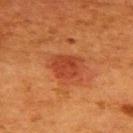The lesion was tiled from a total-body skin photograph and was not biopsied. A lesion tile, about 15 mm wide, cut from a 3D total-body photograph. From the back. The subject is a female aged around 50. The tile uses cross-polarized illumination. Longest diameter approximately 3.5 mm.